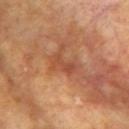This lesion was catalogued during total-body skin photography and was not selected for biopsy. Cropped from a total-body skin-imaging series; the visible field is about 15 mm. The tile uses cross-polarized illumination. A female subject aged approximately 75. The total-body-photography lesion software estimated a lesion color around L≈49 a*≈27 b*≈36 in CIELAB and a lesion-to-skin contrast of about 5.5 (normalized; higher = more distinct). It also reported a border-irregularity rating of about 4/10, internal color variation of about 0.5 on a 0–10 scale, and peripheral color asymmetry of about 0. From the left upper arm. Measured at roughly 3 mm in maximum diameter.